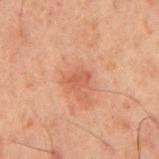Clinical impression: No biopsy was performed on this lesion — it was imaged during a full skin examination and was not determined to be concerning. Clinical summary: The lesion is on the mid back. Imaged with cross-polarized lighting. The subject is a male aged around 60. The lesion-visualizer software estimated a mean CIELAB color near L≈43 a*≈23 b*≈27 and a normalized lesion–skin contrast near 5.5. The software also gave a border-irregularity index near 3/10 and a peripheral color-asymmetry measure near 0.5. A close-up tile cropped from a whole-body skin photograph, about 15 mm across.